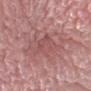biopsy_status: not biopsied; imaged during a skin examination
patient:
  sex: female
  age_approx: 50
image:
  source: total-body photography crop
  field_of_view_mm: 15
site: left lower leg